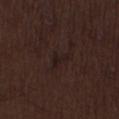Image and clinical context:
Approximately 3 mm at its widest. A male patient aged 68–72. Captured under white-light illumination. A close-up tile cropped from a whole-body skin photograph, about 15 mm across. Located on the left thigh.